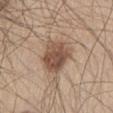biopsy status: catalogued during a skin exam; not biopsied | subject: male, about 60 years old | tile lighting: white-light illumination | anatomic site: the left thigh | lesion diameter: about 4.5 mm | image source: total-body-photography crop, ~15 mm field of view.